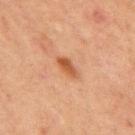The lesion was photographed on a routine skin check and not biopsied; there is no pathology result.
An algorithmic analysis of the crop reported a footprint of about 4 mm², an eccentricity of roughly 0.9, and two-axis asymmetry of about 0.3. It also reported a nevus-likeness score of about 95/100 and lesion-presence confidence of about 100/100.
A male patient, in their mid-60s.
A region of skin cropped from a whole-body photographic capture, roughly 15 mm wide.
Located on the front of the torso.
About 3 mm across.
Captured under cross-polarized illumination.